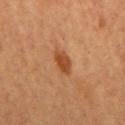Clinical summary: From the right upper arm. A 15 mm close-up extracted from a 3D total-body photography capture. A female subject, about 60 years old.